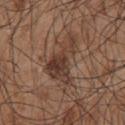<case>
  <biopsy_status>not biopsied; imaged during a skin examination</biopsy_status>
  <lesion_size>
    <long_diameter_mm_approx>7.0</long_diameter_mm_approx>
  </lesion_size>
  <patient>
    <sex>male</sex>
    <age_approx>55</age_approx>
  </patient>
  <image>
    <source>total-body photography crop</source>
    <field_of_view_mm>15</field_of_view_mm>
  </image>
  <site>chest</site>
</case>